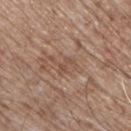Case summary:
– notes · total-body-photography surveillance lesion; no biopsy
– acquisition · ~15 mm crop, total-body skin-cancer survey
– image-analysis metrics · a border-irregularity index near 6/10 and radial color variation of about 0; a nevus-likeness score of about 0/100
– subject · male, aged approximately 65
– body site · the front of the torso